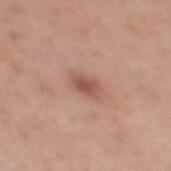{"biopsy_status": "not biopsied; imaged during a skin examination", "patient": {"sex": "female", "age_approx": 35}, "image": {"source": "total-body photography crop", "field_of_view_mm": 15}, "lighting": "white-light", "lesion_size": {"long_diameter_mm_approx": 2.5}, "site": "upper back", "automated_metrics": {"color_variation_0_10": 2.0, "peripheral_color_asymmetry": 0.5, "nevus_likeness_0_100": 90, "lesion_detection_confidence_0_100": 100}}